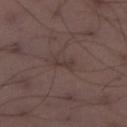An algorithmic analysis of the crop reported a footprint of about 2.5 mm², an outline eccentricity of about 0.9 (0 = round, 1 = elongated), and a symmetry-axis asymmetry near 0.4. And it measured an average lesion color of about L≈35 a*≈14 b*≈17 (CIELAB), a lesion–skin lightness drop of about 6, and a normalized lesion–skin contrast near 5.5. The analysis additionally found border irregularity of about 4.5 on a 0–10 scale, a color-variation rating of about 0/10, and radial color variation of about 0.
A region of skin cropped from a whole-body photographic capture, roughly 15 mm wide.
Located on the right lower leg.
Imaged with white-light lighting.
Longest diameter approximately 2.5 mm.
A male patient aged around 40.